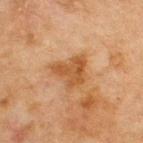{"image": {"source": "total-body photography crop", "field_of_view_mm": 15}, "site": "chest", "lesion_size": {"long_diameter_mm_approx": 4.0}, "patient": {"sex": "male", "age_approx": 65}, "automated_metrics": {"cielab_L": 42, "cielab_a": 20, "cielab_b": 33, "vs_skin_darker_L": 8.0, "vs_skin_contrast_norm": 7.5, "nevus_likeness_0_100": 0}, "lighting": "cross-polarized"}